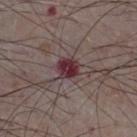Notes:
- workup — no biopsy performed (imaged during a skin exam)
- patient — male, aged 73–77
- lesion diameter — about 2.5 mm
- image — 15 mm crop, total-body photography
- tile lighting — white-light
- anatomic site — the right thigh